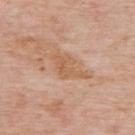| feature | finding |
|---|---|
| workup | imaged on a skin check; not biopsied |
| location | the upper back |
| lesion size | ≈4.5 mm |
| subject | male, aged approximately 75 |
| illumination | white-light |
| image | total-body-photography crop, ~15 mm field of view |